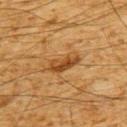This lesion was catalogued during total-body skin photography and was not selected for biopsy.
A lesion tile, about 15 mm wide, cut from a 3D total-body photograph.
Longest diameter approximately 4 mm.
The total-body-photography lesion software estimated an area of roughly 5.5 mm² and a shape-asymmetry score of about 0.25 (0 = symmetric). It also reported a mean CIELAB color near L≈39 a*≈20 b*≈36, about 10 CIELAB-L* units darker than the surrounding skin, and a normalized lesion–skin contrast near 8.5.
The patient is a male aged 58 to 62.
From the upper back.